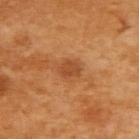This lesion was catalogued during total-body skin photography and was not selected for biopsy. About 2.5 mm across. An algorithmic analysis of the crop reported an eccentricity of roughly 0.65 and a symmetry-axis asymmetry near 0.25. And it measured a mean CIELAB color near L≈49 a*≈27 b*≈41, about 9 CIELAB-L* units darker than the surrounding skin, and a normalized border contrast of about 6.5. The analysis additionally found border irregularity of about 2 on a 0–10 scale, a color-variation rating of about 1.5/10, and peripheral color asymmetry of about 0.5. The lesion is on the upper back. Imaged with cross-polarized lighting. The subject is a female in their mid-50s. Cropped from a total-body skin-imaging series; the visible field is about 15 mm.